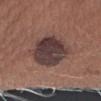No biopsy was performed on this lesion — it was imaged during a full skin examination and was not determined to be concerning. A female patient, aged approximately 25. This image is a 15 mm lesion crop taken from a total-body photograph. The lesion-visualizer software estimated a lesion area of about 22 mm², an outline eccentricity of about 0.75 (0 = round, 1 = elongated), and a symmetry-axis asymmetry near 0.15. It also reported a mean CIELAB color near L≈38 a*≈16 b*≈18, a lesion–skin lightness drop of about 12, and a lesion-to-skin contrast of about 11.5 (normalized; higher = more distinct). And it measured a border-irregularity index near 2/10, internal color variation of about 5 on a 0–10 scale, and radial color variation of about 1.5. It also reported a classifier nevus-likeness of about 0/100 and a lesion-detection confidence of about 100/100. From the right upper arm. The recorded lesion diameter is about 6.5 mm.